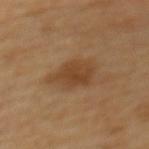The lesion is located on the upper back. A 15 mm close-up extracted from a 3D total-body photography capture. A female patient, about 60 years old.